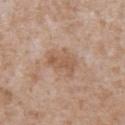The lesion was photographed on a routine skin check and not biopsied; there is no pathology result. Automated image analysis of the tile measured a lesion area of about 10 mm², an outline eccentricity of about 0.75 (0 = round, 1 = elongated), and a symmetry-axis asymmetry near 0.25. It also reported a lesion color around L≈57 a*≈18 b*≈30 in CIELAB, about 8 CIELAB-L* units darker than the surrounding skin, and a lesion-to-skin contrast of about 6 (normalized; higher = more distinct). The analysis additionally found a classifier nevus-likeness of about 0/100 and a detector confidence of about 100 out of 100 that the crop contains a lesion. Cropped from a total-body skin-imaging series; the visible field is about 15 mm. The lesion's longest dimension is about 4.5 mm. From the chest. This is a white-light tile. A male patient, aged 63 to 67.